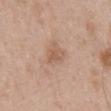{
  "biopsy_status": "not biopsied; imaged during a skin examination",
  "site": "mid back",
  "patient": {
    "sex": "male",
    "age_approx": 50
  },
  "image": {
    "source": "total-body photography crop",
    "field_of_view_mm": 15
  }
}